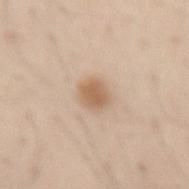Case summary:
– imaging modality · ~15 mm crop, total-body skin-cancer survey
– TBP lesion metrics · a shape eccentricity near 0.55 and a symmetry-axis asymmetry near 0.2; a mean CIELAB color near L≈61 a*≈17 b*≈32, about 11 CIELAB-L* units darker than the surrounding skin, and a normalized lesion–skin contrast near 7.5; a border-irregularity index near 1.5/10, internal color variation of about 2 on a 0–10 scale, and peripheral color asymmetry of about 0.5; a nevus-likeness score of about 95/100 and a lesion-detection confidence of about 100/100
– lesion size · ≈2.5 mm
– anatomic site · the lower back
– subject · male, about 60 years old